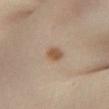This lesion was catalogued during total-body skin photography and was not selected for biopsy.
A close-up tile cropped from a whole-body skin photograph, about 15 mm across.
The subject is a female aged 38 to 42.
The lesion's longest dimension is about 2.5 mm.
The lesion is located on the left lower leg.
An algorithmic analysis of the crop reported an average lesion color of about L≈54 a*≈17 b*≈31 (CIELAB) and about 10 CIELAB-L* units darker than the surrounding skin. And it measured a border-irregularity rating of about 1.5/10 and a peripheral color-asymmetry measure near 1. The analysis additionally found a classifier nevus-likeness of about 95/100 and lesion-presence confidence of about 100/100.